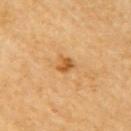Part of a total-body skin-imaging series; this lesion was reviewed on a skin check and was not flagged for biopsy. The lesion is on the right upper arm. The tile uses cross-polarized illumination. A female patient aged 68–72. Cropped from a whole-body photographic skin survey; the tile spans about 15 mm. The total-body-photography lesion software estimated a lesion area of about 3 mm² and an outline eccentricity of about 0.6 (0 = round, 1 = elongated). The software also gave an average lesion color of about L≈58 a*≈24 b*≈47 (CIELAB), roughly 12 lightness units darker than nearby skin, and a normalized border contrast of about 8. It also reported a border-irregularity rating of about 2.5/10, a color-variation rating of about 2/10, and a peripheral color-asymmetry measure near 1. It also reported an automated nevus-likeness rating near 65 out of 100 and lesion-presence confidence of about 100/100. Approximately 2 mm at its widest.